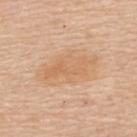No biopsy was performed on this lesion — it was imaged during a full skin examination and was not determined to be concerning. The subject is a female in their mid- to late 60s. Cropped from a total-body skin-imaging series; the visible field is about 15 mm. On the upper back.